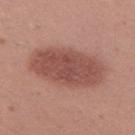follow-up=total-body-photography surveillance lesion; no biopsy
anatomic site=the leg
subject=female, approximately 30 years of age
acquisition=~15 mm crop, total-body skin-cancer survey